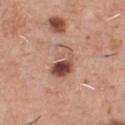<case>
  <biopsy_status>not biopsied; imaged during a skin examination</biopsy_status>
  <automated_metrics>
    <area_mm2_approx>11.0</area_mm2_approx>
    <eccentricity>0.8</eccentricity>
    <shape_asymmetry>0.3</shape_asymmetry>
    <nevus_likeness_0_100>90</nevus_likeness_0_100>
    <lesion_detection_confidence_0_100>100</lesion_detection_confidence_0_100>
  </automated_metrics>
  <image>
    <source>total-body photography crop</source>
    <field_of_view_mm>15</field_of_view_mm>
  </image>
  <patient>
    <sex>male</sex>
    <age_approx>45</age_approx>
  </patient>
  <site>chest</site>
  <lighting>white-light</lighting>
  <lesion_size>
    <long_diameter_mm_approx>4.5</long_diameter_mm_approx>
  </lesion_size>
</case>